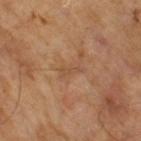The lesion was tiled from a total-body skin photograph and was not biopsied.
A male subject roughly 65 years of age.
This is a cross-polarized tile.
Longest diameter approximately 3 mm.
Cropped from a total-body skin-imaging series; the visible field is about 15 mm.